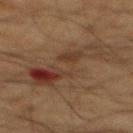Notes:
* workup: catalogued during a skin exam; not biopsied
* subject: male, in their mid-60s
* anatomic site: the mid back
* image source: 15 mm crop, total-body photography
* illumination: cross-polarized illumination
* lesion diameter: about 10.5 mm